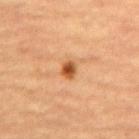Assessment:
No biopsy was performed on this lesion — it was imaged during a full skin examination and was not determined to be concerning.
Background:
A region of skin cropped from a whole-body photographic capture, roughly 15 mm wide. Measured at roughly 2 mm in maximum diameter. This is a cross-polarized tile. A male subject, about 65 years old. From the back.